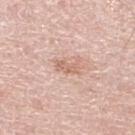* notes — imaged on a skin check; not biopsied
* diameter — about 2.5 mm
* image — total-body-photography crop, ~15 mm field of view
* subject — male, in their 70s
* image-analysis metrics — internal color variation of about 2 on a 0–10 scale and a peripheral color-asymmetry measure near 0.5
* location — the right lower leg
* illumination — white-light